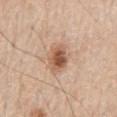Recorded during total-body skin imaging; not selected for excision or biopsy.
The lesion's longest dimension is about 3.5 mm.
The tile uses white-light illumination.
A male patient, aged 58 to 62.
The lesion-visualizer software estimated a lesion area of about 7 mm², a shape eccentricity near 0.7, and a symmetry-axis asymmetry near 0.25. It also reported about 14 CIELAB-L* units darker than the surrounding skin and a lesion-to-skin contrast of about 9 (normalized; higher = more distinct). It also reported a detector confidence of about 100 out of 100 that the crop contains a lesion.
The lesion is on the back.
This image is a 15 mm lesion crop taken from a total-body photograph.